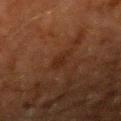Q: Is there a histopathology result?
A: imaged on a skin check; not biopsied
Q: Lesion location?
A: the right upper arm
Q: Patient demographics?
A: male, about 60 years old
Q: What kind of image is this?
A: ~15 mm crop, total-body skin-cancer survey